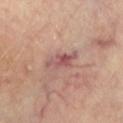Part of a total-body skin-imaging series; this lesion was reviewed on a skin check and was not flagged for biopsy.
The lesion-visualizer software estimated border irregularity of about 4.5 on a 0–10 scale, a color-variation rating of about 6/10, and radial color variation of about 2. The analysis additionally found an automated nevus-likeness rating near 0 out of 100 and lesion-presence confidence of about 95/100.
The subject is a female aged 63–67.
Longest diameter approximately 4 mm.
Captured under cross-polarized illumination.
A 15 mm crop from a total-body photograph taken for skin-cancer surveillance.
The lesion is located on the left lower leg.